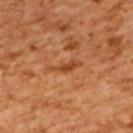This lesion was catalogued during total-body skin photography and was not selected for biopsy. The patient is a female about 55 years old. Located on the upper back. Imaged with cross-polarized lighting. Longest diameter approximately 4 mm. A lesion tile, about 15 mm wide, cut from a 3D total-body photograph.